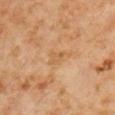Case summary:
- follow-up — imaged on a skin check; not biopsied